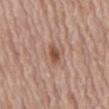| field | value |
|---|---|
| biopsy status | catalogued during a skin exam; not biopsied |
| diameter | ≈3 mm |
| imaging modality | total-body-photography crop, ~15 mm field of view |
| tile lighting | white-light |
| site | the abdomen |
| patient | male, in their 70s |